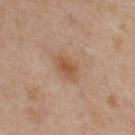notes: catalogued during a skin exam; not biopsied | imaging modality: 15 mm crop, total-body photography | automated lesion analysis: a lesion color around L≈50 a*≈19 b*≈31 in CIELAB, a lesion–skin lightness drop of about 8, and a lesion-to-skin contrast of about 7 (normalized; higher = more distinct) | illumination: cross-polarized illumination | lesion diameter: about 3.5 mm | subject: female, about 50 years old | body site: the left upper arm.